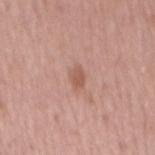Assessment: The lesion was tiled from a total-body skin photograph and was not biopsied. Clinical summary: Measured at roughly 2.5 mm in maximum diameter. Imaged with white-light lighting. The patient is a male in their mid- to late 60s. Cropped from a total-body skin-imaging series; the visible field is about 15 mm. From the mid back.